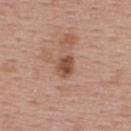biopsy status: imaged on a skin check; not biopsied | lesion diameter: ≈2.5 mm | image: ~15 mm tile from a whole-body skin photo | subject: female, about 55 years old | automated lesion analysis: an average lesion color of about L≈49 a*≈23 b*≈29 (CIELAB); border irregularity of about 2 on a 0–10 scale, internal color variation of about 2.5 on a 0–10 scale, and radial color variation of about 1; an automated nevus-likeness rating near 40 out of 100 and lesion-presence confidence of about 100/100 | tile lighting: white-light | location: the upper back.